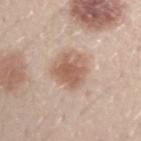Assessment:
Part of a total-body skin-imaging series; this lesion was reviewed on a skin check and was not flagged for biopsy.
Clinical summary:
The patient is a male aged around 30. Measured at roughly 4.5 mm in maximum diameter. Captured under white-light illumination. On the left forearm. Cropped from a whole-body photographic skin survey; the tile spans about 15 mm.